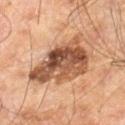Findings:
– biopsy status — imaged on a skin check; not biopsied
– lesion diameter — ≈7 mm
– image-analysis metrics — roughly 16 lightness units darker than nearby skin; a classifier nevus-likeness of about 15/100 and lesion-presence confidence of about 100/100
– image — ~15 mm tile from a whole-body skin photo
– illumination — cross-polarized
– location — the right leg
– subject — male, approximately 60 years of age